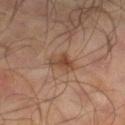Assessment:
Imaged during a routine full-body skin examination; the lesion was not biopsied and no histopathology is available.
Clinical summary:
Measured at roughly 3 mm in maximum diameter. A male subject, in their mid-60s. An algorithmic analysis of the crop reported a border-irregularity index near 2.5/10, internal color variation of about 4 on a 0–10 scale, and peripheral color asymmetry of about 1.5. It also reported an automated nevus-likeness rating near 30 out of 100 and a detector confidence of about 100 out of 100 that the crop contains a lesion. The tile uses cross-polarized illumination. On the right lower leg. A close-up tile cropped from a whole-body skin photograph, about 15 mm across.